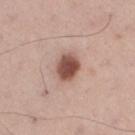notes = no biopsy performed (imaged during a skin exam) | body site = the right thigh | lesion diameter = ≈3.5 mm | image = total-body-photography crop, ~15 mm field of view | subject = male, in their mid- to late 50s.